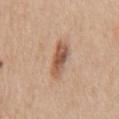Captured during whole-body skin photography for melanoma surveillance; the lesion was not biopsied. An algorithmic analysis of the crop reported a footprint of about 7.5 mm² and a shape eccentricity near 0.85. The software also gave an average lesion color of about L≈55 a*≈21 b*≈31 (CIELAB), a lesion–skin lightness drop of about 13, and a normalized border contrast of about 9. It also reported a lesion-detection confidence of about 100/100. Imaged with white-light lighting. A male subject about 60 years old. The lesion is located on the back. This image is a 15 mm lesion crop taken from a total-body photograph. The recorded lesion diameter is about 4 mm.